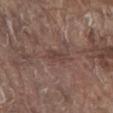Acquisition and patient details: Approximately 2.5 mm at its widest. The tile uses white-light illumination. On the front of the torso. This image is a 15 mm lesion crop taken from a total-body photograph. A male patient, aged 78 to 82.